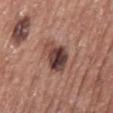Clinical impression:
Captured during whole-body skin photography for melanoma surveillance; the lesion was not biopsied.
Background:
Cropped from a total-body skin-imaging series; the visible field is about 15 mm. This is a white-light tile. The subject is a male in their mid-70s. The lesion is located on the back. The lesion-visualizer software estimated an eccentricity of roughly 0.8 and two-axis asymmetry of about 0.25. The analysis additionally found a mean CIELAB color near L≈42 a*≈20 b*≈22, a lesion–skin lightness drop of about 15, and a normalized lesion–skin contrast near 11.5. And it measured a nevus-likeness score of about 45/100.